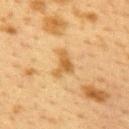Recorded during total-body skin imaging; not selected for excision or biopsy.
The tile uses cross-polarized illumination.
A roughly 15 mm field-of-view crop from a total-body skin photograph.
The lesion is located on the upper back.
Longest diameter approximately 3.5 mm.
A female subject, approximately 40 years of age.